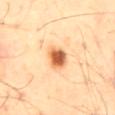Impression: The lesion was photographed on a routine skin check and not biopsied; there is no pathology result. Clinical summary: An algorithmic analysis of the crop reported an area of roughly 6 mm², an outline eccentricity of about 0.7 (0 = round, 1 = elongated), and a shape-asymmetry score of about 0.2 (0 = symmetric). It also reported a mean CIELAB color near L≈66 a*≈28 b*≈44, roughly 20 lightness units darker than nearby skin, and a normalized border contrast of about 11. The analysis additionally found border irregularity of about 2 on a 0–10 scale, a color-variation rating of about 5.5/10, and peripheral color asymmetry of about 1.5. The lesion is located on the mid back. About 3 mm across. A male patient, approximately 40 years of age. A region of skin cropped from a whole-body photographic capture, roughly 15 mm wide. Captured under cross-polarized illumination.